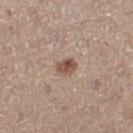Impression:
Captured during whole-body skin photography for melanoma surveillance; the lesion was not biopsied.
Acquisition and patient details:
The total-body-photography lesion software estimated an average lesion color of about L≈51 a*≈18 b*≈27 (CIELAB), a lesion–skin lightness drop of about 12, and a normalized lesion–skin contrast near 8.5. The lesion's longest dimension is about 2.5 mm. A region of skin cropped from a whole-body photographic capture, roughly 15 mm wide. Imaged with white-light lighting. A female patient, roughly 50 years of age. Located on the left thigh.